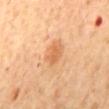workup: imaged on a skin check; not biopsied.